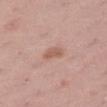Q: Was this lesion biopsied?
A: no biopsy performed (imaged during a skin exam)
Q: What is the imaging modality?
A: ~15 mm tile from a whole-body skin photo
Q: Who is the patient?
A: female, in their mid- to late 40s
Q: What is the lesion's diameter?
A: ≈2.5 mm
Q: What is the anatomic site?
A: the right thigh
Q: What lighting was used for the tile?
A: white-light illumination